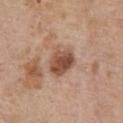The lesion was photographed on a routine skin check and not biopsied; there is no pathology result.
The lesion is on the chest.
Longest diameter approximately 4 mm.
A close-up tile cropped from a whole-body skin photograph, about 15 mm across.
The subject is a female aged 73 to 77.